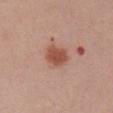Case summary:
- lighting — white-light
- lesion size — ~3 mm (longest diameter)
- image-analysis metrics — a border-irregularity rating of about 2/10, a within-lesion color-variation index near 2/10, and radial color variation of about 0.5; lesion-presence confidence of about 100/100
- image — total-body-photography crop, ~15 mm field of view
- subject — male, in their 40s
- anatomic site — the arm